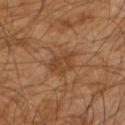The lesion is located on the right arm.
This image is a 15 mm lesion crop taken from a total-body photograph.
A male patient aged approximately 60.